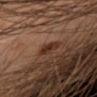Findings:
- notes · no biopsy performed (imaged during a skin exam)
- size · ~2.5 mm (longest diameter)
- image · total-body-photography crop, ~15 mm field of view
- body site · the left arm
- patient · male, aged 48 to 52